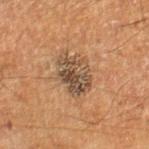No biopsy was performed on this lesion — it was imaged during a full skin examination and was not determined to be concerning.
A male subject, about 65 years old.
This is a cross-polarized tile.
The recorded lesion diameter is about 5 mm.
The lesion is located on the right lower leg.
A 15 mm close-up extracted from a 3D total-body photography capture.